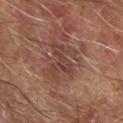imaging modality=15 mm crop, total-body photography | lesion diameter=~5 mm (longest diameter) | lighting=cross-polarized | body site=the right forearm | subject=male, aged approximately 70.